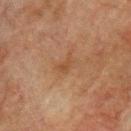Recorded during total-body skin imaging; not selected for excision or biopsy.
About 2.5 mm across.
A male patient aged 73 to 77.
The lesion-visualizer software estimated a shape eccentricity near 0.85 and a symmetry-axis asymmetry near 0.55. It also reported about 5 CIELAB-L* units darker than the surrounding skin and a normalized lesion–skin contrast near 5.5. The software also gave a border-irregularity index near 5.5/10, a color-variation rating of about 0.5/10, and peripheral color asymmetry of about 0. It also reported an automated nevus-likeness rating near 0 out of 100.
The lesion is on the front of the torso.
A 15 mm close-up extracted from a 3D total-body photography capture.
Imaged with cross-polarized lighting.